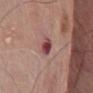Imaged during a routine full-body skin examination; the lesion was not biopsied and no histopathology is available.
The patient is a male aged 78 to 82.
From the chest.
A lesion tile, about 15 mm wide, cut from a 3D total-body photograph.
The recorded lesion diameter is about 2.5 mm.
Imaged with white-light lighting.